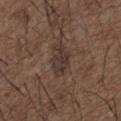{
  "biopsy_status": "not biopsied; imaged during a skin examination",
  "lighting": "white-light",
  "image": {
    "source": "total-body photography crop",
    "field_of_view_mm": 15
  },
  "site": "back",
  "patient": {
    "sex": "male",
    "age_approx": 50
  },
  "lesion_size": {
    "long_diameter_mm_approx": 3.5
  }
}